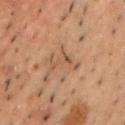Assessment: Imaged during a routine full-body skin examination; the lesion was not biopsied and no histopathology is available. Background: The recorded lesion diameter is about 4 mm. The lesion is located on the chest. The patient is a male about 50 years old. Captured under cross-polarized illumination. A region of skin cropped from a whole-body photographic capture, roughly 15 mm wide.